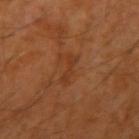The lesion was tiled from a total-body skin photograph and was not biopsied.
The lesion is located on the right upper arm.
A male subject, roughly 60 years of age.
Captured under cross-polarized illumination.
The recorded lesion diameter is about 3 mm.
A 15 mm close-up tile from a total-body photography series done for melanoma screening.